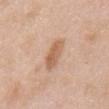<lesion>
  <biopsy_status>not biopsied; imaged during a skin examination</biopsy_status>
  <image>
    <source>total-body photography crop</source>
    <field_of_view_mm>15</field_of_view_mm>
  </image>
  <lighting>white-light</lighting>
  <automated_metrics>
    <area_mm2_approx>7.5</area_mm2_approx>
    <eccentricity>0.9</eccentricity>
    <shape_asymmetry>0.2</shape_asymmetry>
    <nevus_likeness_0_100>30</nevus_likeness_0_100>
    <lesion_detection_confidence_0_100>100</lesion_detection_confidence_0_100>
  </automated_metrics>
  <site>abdomen</site>
  <patient>
    <sex>male</sex>
    <age_approx>60</age_approx>
  </patient>
</lesion>